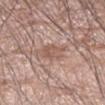- notes: imaged on a skin check; not biopsied
- illumination: white-light
- acquisition: ~15 mm crop, total-body skin-cancer survey
- location: the arm
- patient: male, aged around 65
- lesion size: about 3.5 mm